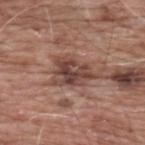biopsy_status: not biopsied; imaged during a skin examination
site: upper back
patient:
  sex: male
  age_approx: 60
lesion_size:
  long_diameter_mm_approx: 5.5
automated_metrics:
  area_mm2_approx: 12.0
  eccentricity: 0.75
  shape_asymmetry: 0.35
  border_irregularity_0_10: 4.5
  color_variation_0_10: 7.0
  peripheral_color_asymmetry: 2.5
image:
  source: total-body photography crop
  field_of_view_mm: 15